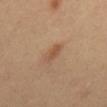Findings:
* biopsy status: no biopsy performed (imaged during a skin exam)
* patient: male, aged 38 to 42
* anatomic site: the back
* image source: ~15 mm tile from a whole-body skin photo